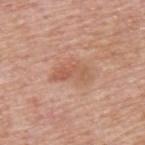Notes:
• notes · total-body-photography surveillance lesion; no biopsy
• automated metrics · an average lesion color of about L≈57 a*≈23 b*≈31 (CIELAB), roughly 8 lightness units darker than nearby skin, and a normalized lesion–skin contrast near 5.5; radial color variation of about 1.5; a classifier nevus-likeness of about 10/100
• size · ~4 mm (longest diameter)
• illumination · white-light illumination
• patient · male, aged around 75
• body site · the upper back
• image source · ~15 mm crop, total-body skin-cancer survey